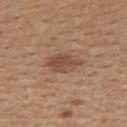{
  "biopsy_status": "not biopsied; imaged during a skin examination",
  "lesion_size": {
    "long_diameter_mm_approx": 4.5
  },
  "automated_metrics": {
    "cielab_L": 48,
    "cielab_a": 19,
    "cielab_b": 29,
    "vs_skin_darker_L": 9.0,
    "vs_skin_contrast_norm": 7.0,
    "border_irregularity_0_10": 2.0,
    "color_variation_0_10": 3.5,
    "peripheral_color_asymmetry": 1.0,
    "nevus_likeness_0_100": 55,
    "lesion_detection_confidence_0_100": 100
  },
  "site": "upper back",
  "patient": {
    "sex": "female",
    "age_approx": 45
  },
  "image": {
    "source": "total-body photography crop",
    "field_of_view_mm": 15
  }
}